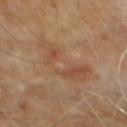Recorded during total-body skin imaging; not selected for excision or biopsy.
Measured at roughly 7 mm in maximum diameter.
On the abdomen.
A 15 mm close-up tile from a total-body photography series done for melanoma screening.
The total-body-photography lesion software estimated a lesion color around L≈47 a*≈18 b*≈30 in CIELAB, a lesion–skin lightness drop of about 6, and a normalized border contrast of about 5. And it measured a nevus-likeness score of about 0/100 and a detector confidence of about 100 out of 100 that the crop contains a lesion.
This is a cross-polarized tile.
The patient is a male roughly 60 years of age.